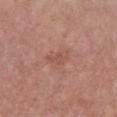Part of a total-body skin-imaging series; this lesion was reviewed on a skin check and was not flagged for biopsy.
A lesion tile, about 15 mm wide, cut from a 3D total-body photograph.
The tile uses white-light illumination.
A female patient, aged approximately 45.
Located on the front of the torso.